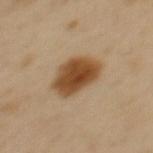follow-up: no biopsy performed (imaged during a skin exam)
patient: female, about 40 years old
lighting: cross-polarized
image-analysis metrics: a mean CIELAB color near L≈47 a*≈19 b*≈35, a lesion–skin lightness drop of about 15, and a lesion-to-skin contrast of about 11.5 (normalized; higher = more distinct); border irregularity of about 2 on a 0–10 scale, a within-lesion color-variation index near 4.5/10, and radial color variation of about 1.5
acquisition: 15 mm crop, total-body photography
anatomic site: the upper back
lesion size: about 5 mm